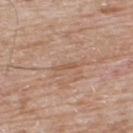Clinical impression:
Imaged during a routine full-body skin examination; the lesion was not biopsied and no histopathology is available.
Background:
The subject is a male approximately 65 years of age. On the upper back. About 2.5 mm across. Imaged with white-light lighting. A 15 mm close-up extracted from a 3D total-body photography capture. An algorithmic analysis of the crop reported a footprint of about 2 mm² and a shape eccentricity near 0.95. And it measured a border-irregularity rating of about 5/10, a color-variation rating of about 0/10, and peripheral color asymmetry of about 0. It also reported a nevus-likeness score of about 0/100 and a detector confidence of about 70 out of 100 that the crop contains a lesion.